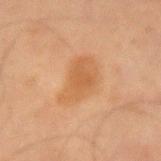Findings:
- workup — total-body-photography surveillance lesion; no biopsy
- automated lesion analysis — an area of roughly 12 mm², a shape eccentricity near 0.85, and two-axis asymmetry of about 0.3; a mean CIELAB color near L≈48 a*≈19 b*≈34 and a normalized lesion–skin contrast near 6; a border-irregularity index near 3.5/10 and a within-lesion color-variation index near 2/10; a nevus-likeness score of about 30/100 and a detector confidence of about 100 out of 100 that the crop contains a lesion
- image source — ~15 mm crop, total-body skin-cancer survey
- diameter — about 5 mm
- subject — male, in their mid-60s
- tile lighting — cross-polarized illumination
- site — the arm